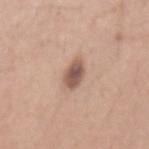Impression:
The lesion was photographed on a routine skin check and not biopsied; there is no pathology result.
Context:
A 15 mm close-up extracted from a 3D total-body photography capture. The lesion is on the mid back. A male subject, aged around 30.